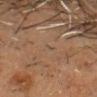Findings:
- biopsy status · imaged on a skin check; not biopsied
- image source · ~15 mm tile from a whole-body skin photo
- body site · the head or neck
- patient · male, aged 48–52